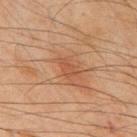No biopsy was performed on this lesion — it was imaged during a full skin examination and was not determined to be concerning. An algorithmic analysis of the crop reported an outline eccentricity of about 0.8 (0 = round, 1 = elongated) and two-axis asymmetry of about 0.35. The software also gave about 5 CIELAB-L* units darker than the surrounding skin and a lesion-to-skin contrast of about 4.5 (normalized; higher = more distinct). The analysis additionally found an automated nevus-likeness rating near 40 out of 100 and lesion-presence confidence of about 100/100. Measured at roughly 2.5 mm in maximum diameter. Captured under cross-polarized illumination. From the mid back. The subject is a male about 45 years old. A roughly 15 mm field-of-view crop from a total-body skin photograph.